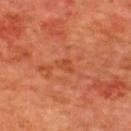A male patient, aged around 65.
On the upper back.
About 2.5 mm across.
A 15 mm crop from a total-body photograph taken for skin-cancer surveillance.
The lesion-visualizer software estimated a shape eccentricity near 0.85 and two-axis asymmetry of about 0.4. The software also gave internal color variation of about 2 on a 0–10 scale and peripheral color asymmetry of about 0.5. And it measured a nevus-likeness score of about 0/100 and a lesion-detection confidence of about 100/100.